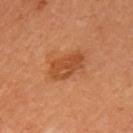acquisition=total-body-photography crop, ~15 mm field of view | automated metrics=a border-irregularity rating of about 2.5/10, internal color variation of about 3 on a 0–10 scale, and peripheral color asymmetry of about 1; a classifier nevus-likeness of about 80/100 and a lesion-detection confidence of about 100/100 | site=the left upper arm | patient=female, aged 33 to 37.